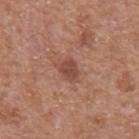The lesion was tiled from a total-body skin photograph and was not biopsied.
A close-up tile cropped from a whole-body skin photograph, about 15 mm across.
The subject is a male in their mid- to late 60s.
On the abdomen.
The recorded lesion diameter is about 2.5 mm.
Automated image analysis of the tile measured a nevus-likeness score of about 70/100 and lesion-presence confidence of about 100/100.
The tile uses white-light illumination.